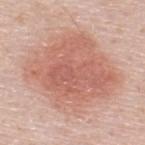Recorded during total-body skin imaging; not selected for excision or biopsy. This is a white-light tile. A male patient, roughly 50 years of age. The lesion's longest dimension is about 10 mm. The lesion is on the upper back. A region of skin cropped from a whole-body photographic capture, roughly 15 mm wide.